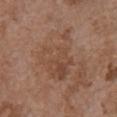| field | value |
|---|---|
| notes | total-body-photography surveillance lesion; no biopsy |
| image source | ~15 mm crop, total-body skin-cancer survey |
| location | the chest |
| lighting | white-light illumination |
| subject | female, in their mid-60s |
| lesion diameter | ~6.5 mm (longest diameter) |